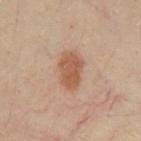notes = imaged on a skin check; not biopsied.